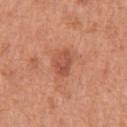Q: Is there a histopathology result?
A: imaged on a skin check; not biopsied